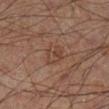Q: Was this lesion biopsied?
A: no biopsy performed (imaged during a skin exam)
Q: What is the imaging modality?
A: ~15 mm crop, total-body skin-cancer survey
Q: What lighting was used for the tile?
A: cross-polarized
Q: What are the patient's age and sex?
A: male, approximately 65 years of age
Q: Lesion size?
A: about 3 mm
Q: What is the anatomic site?
A: the right lower leg
Q: Automated lesion metrics?
A: an area of roughly 5.5 mm², an eccentricity of roughly 0.65, and a shape-asymmetry score of about 0.15 (0 = symmetric); border irregularity of about 1.5 on a 0–10 scale and internal color variation of about 3.5 on a 0–10 scale; an automated nevus-likeness rating near 0 out of 100 and lesion-presence confidence of about 100/100